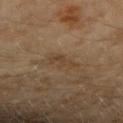The lesion was tiled from a total-body skin photograph and was not biopsied. The subject is a female aged 58 to 62. About 3 mm across. From the right forearm. A roughly 15 mm field-of-view crop from a total-body skin photograph.